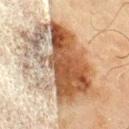– notes · total-body-photography surveillance lesion; no biopsy
– TBP lesion metrics · an automated nevus-likeness rating near 95 out of 100 and a lesion-detection confidence of about 100/100
– subject · male, approximately 70 years of age
– tile lighting · cross-polarized illumination
– image source · total-body-photography crop, ~15 mm field of view
– body site · the chest
– lesion diameter · about 12 mm